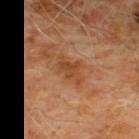Context: On the front of the torso. The tile uses cross-polarized illumination. A close-up tile cropped from a whole-body skin photograph, about 15 mm across. The subject is a male in their 60s. Automated image analysis of the tile measured a lesion area of about 5.5 mm², an eccentricity of roughly 0.8, and a shape-asymmetry score of about 0.35 (0 = symmetric). It also reported about 8 CIELAB-L* units darker than the surrounding skin and a normalized border contrast of about 6.5. The software also gave a border-irregularity index near 4.5/10, a color-variation rating of about 2/10, and radial color variation of about 0.5.